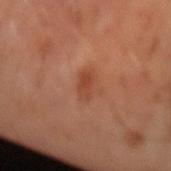Clinical impression:
Captured during whole-body skin photography for melanoma surveillance; the lesion was not biopsied.
Image and clinical context:
A male patient aged around 65. Cropped from a whole-body photographic skin survey; the tile spans about 15 mm. This is a cross-polarized tile.